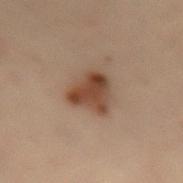Q: Illumination type?
A: cross-polarized
Q: Patient demographics?
A: female, aged around 30
Q: Where on the body is the lesion?
A: the lower back
Q: How large is the lesion?
A: ≈4.5 mm
Q: Automated lesion metrics?
A: an average lesion color of about L≈35 a*≈16 b*≈24 (CIELAB), roughly 10 lightness units darker than nearby skin, and a normalized lesion–skin contrast near 9.5
Q: What is the imaging modality?
A: ~15 mm crop, total-body skin-cancer survey